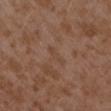Impression:
No biopsy was performed on this lesion — it was imaged during a full skin examination and was not determined to be concerning.
Image and clinical context:
This is a white-light tile. The lesion is located on the left forearm. Cropped from a total-body skin-imaging series; the visible field is about 15 mm. A female subject, aged approximately 35. Longest diameter approximately 3 mm.